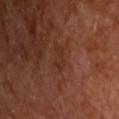| key | value |
|---|---|
| imaging modality | total-body-photography crop, ~15 mm field of view |
| illumination | cross-polarized illumination |
| lesion size | ~2.5 mm (longest diameter) |
| location | the front of the torso |
| patient | male, aged approximately 65 |